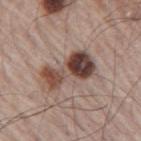<case>
  <biopsy_status>not biopsied; imaged during a skin examination</biopsy_status>
  <image>
    <source>total-body photography crop</source>
    <field_of_view_mm>15</field_of_view_mm>
  </image>
  <site>right upper arm</site>
  <patient>
    <sex>male</sex>
    <age_approx>65</age_approx>
  </patient>
  <lighting>white-light</lighting>
  <automated_metrics>
    <area_mm2_approx>14.0</area_mm2_approx>
    <eccentricity>0.9</eccentricity>
    <shape_asymmetry>0.35</shape_asymmetry>
    <border_irregularity_0_10>4.5</border_irregularity_0_10>
    <color_variation_0_10>10.0</color_variation_0_10>
    <peripheral_color_asymmetry>5.0</peripheral_color_asymmetry>
  </automated_metrics>
</case>